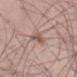Assessment:
The lesion was tiled from a total-body skin photograph and was not biopsied.
Background:
A male subject about 50 years old. A roughly 15 mm field-of-view crop from a total-body skin photograph. The lesion is located on the left thigh.